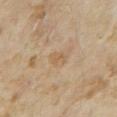biopsy_status: not biopsied; imaged during a skin examination
patient:
  sex: female
  age_approx: 35
automated_metrics:
  border_irregularity_0_10: 4.0
  color_variation_0_10: 0.5
  peripheral_color_asymmetry: 0.0
  nevus_likeness_0_100: 0
  lesion_detection_confidence_0_100: 100
image:
  source: total-body photography crop
  field_of_view_mm: 15
site: right forearm
lesion_size:
  long_diameter_mm_approx: 2.5